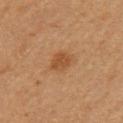Recorded during total-body skin imaging; not selected for excision or biopsy. About 2.5 mm across. The subject is a female aged around 50. A roughly 15 mm field-of-view crop from a total-body skin photograph. Located on the left upper arm. Automated tile analysis of the lesion measured a border-irregularity rating of about 2/10, a within-lesion color-variation index near 2/10, and peripheral color asymmetry of about 1. It also reported a nevus-likeness score of about 50/100.